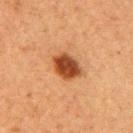biopsy status=imaged on a skin check; not biopsied | imaging modality=total-body-photography crop, ~15 mm field of view | body site=the right upper arm | illumination=cross-polarized illumination | subject=female, aged around 60.